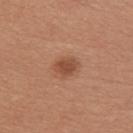| key | value |
|---|---|
| notes | no biopsy performed (imaged during a skin exam) |
| body site | the right upper arm |
| image | ~15 mm tile from a whole-body skin photo |
| subject | female, aged 23–27 |